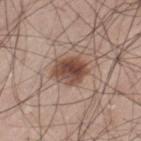Impression: No biopsy was performed on this lesion — it was imaged during a full skin examination and was not determined to be concerning. Context: A lesion tile, about 15 mm wide, cut from a 3D total-body photograph. The lesion-visualizer software estimated an area of roughly 9.5 mm² and a symmetry-axis asymmetry near 0.2. The analysis additionally found a border-irregularity rating of about 2/10 and a color-variation rating of about 6/10. The software also gave a nevus-likeness score of about 95/100. A male subject in their 70s. Imaged with white-light lighting. Located on the lower back.